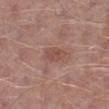Q: Is there a histopathology result?
A: total-body-photography surveillance lesion; no biopsy
Q: Who is the patient?
A: male, aged approximately 55
Q: Lesion location?
A: the right lower leg
Q: What kind of image is this?
A: 15 mm crop, total-body photography